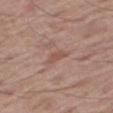This lesion was catalogued during total-body skin photography and was not selected for biopsy. Located on the right thigh. The recorded lesion diameter is about 3 mm. The subject is a male aged 68 to 72. This image is a 15 mm lesion crop taken from a total-body photograph.